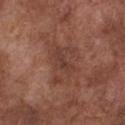  biopsy_status: not biopsied; imaged during a skin examination
  lighting: white-light
  automated_metrics:
    area_mm2_approx: 13.0
    eccentricity: 0.7
    shape_asymmetry: 0.4
    cielab_L: 40
    cielab_a: 21
    cielab_b: 26
    vs_skin_darker_L: 7.0
    vs_skin_contrast_norm: 6.0
    border_irregularity_0_10: 5.5
    color_variation_0_10: 3.0
    nevus_likeness_0_100: 0
    lesion_detection_confidence_0_100: 100
  patient:
    sex: male
    age_approx: 75
  image:
    source: total-body photography crop
    field_of_view_mm: 15
  site: chest
  lesion_size:
    long_diameter_mm_approx: 5.0